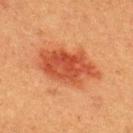<lesion>
<biopsy_status>not biopsied; imaged during a skin examination</biopsy_status>
<automated_metrics>
  <eccentricity>0.85</eccentricity>
  <nevus_likeness_0_100>100</nevus_likeness_0_100>
</automated_metrics>
<image>
  <source>total-body photography crop</source>
  <field_of_view_mm>15</field_of_view_mm>
</image>
<patient>
  <sex>male</sex>
  <age_approx>40</age_approx>
</patient>
<site>upper back</site>
<lighting>cross-polarized</lighting>
</lesion>